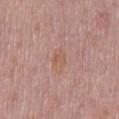Clinical impression:
The lesion was tiled from a total-body skin photograph and was not biopsied.